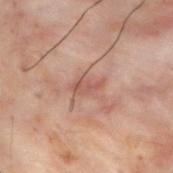Impression:
Imaged during a routine full-body skin examination; the lesion was not biopsied and no histopathology is available.
Acquisition and patient details:
A close-up tile cropped from a whole-body skin photograph, about 15 mm across. The lesion is located on the upper back. Longest diameter approximately 3 mm. The tile uses cross-polarized illumination. A male subject, aged 28 to 32.